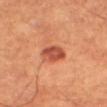Captured during whole-body skin photography for melanoma surveillance; the lesion was not biopsied. A male patient aged around 60. The lesion is located on the right thigh. A roughly 15 mm field-of-view crop from a total-body skin photograph. Imaged with cross-polarized lighting.